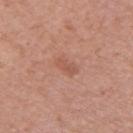Q: Was this lesion biopsied?
A: total-body-photography surveillance lesion; no biopsy
Q: What is the lesion's diameter?
A: ≈2.5 mm
Q: What is the imaging modality?
A: ~15 mm tile from a whole-body skin photo
Q: Patient demographics?
A: female, aged 58–62
Q: Lesion location?
A: the arm
Q: What lighting was used for the tile?
A: white-light illumination